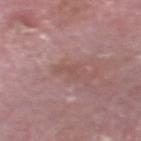Recorded during total-body skin imaging; not selected for excision or biopsy. About 2.5 mm across. The lesion is on the head or neck. A lesion tile, about 15 mm wide, cut from a 3D total-body photograph. The lesion-visualizer software estimated a mean CIELAB color near L≈51 a*≈20 b*≈23 and a normalized border contrast of about 4.5. The software also gave a classifier nevus-likeness of about 0/100 and a detector confidence of about 100 out of 100 that the crop contains a lesion. The subject is a male aged 63–67.